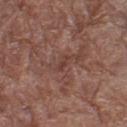biopsy status=total-body-photography surveillance lesion; no biopsy.